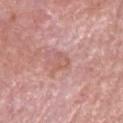<record>
  <biopsy_status>not biopsied; imaged during a skin examination</biopsy_status>
  <patient>
    <sex>male</sex>
    <age_approx>75</age_approx>
  </patient>
  <image>
    <source>total-body photography crop</source>
    <field_of_view_mm>15</field_of_view_mm>
  </image>
  <site>head or neck</site>
  <lesion_size>
    <long_diameter_mm_approx>3.0</long_diameter_mm_approx>
  </lesion_size>
  <lighting>white-light</lighting>
</record>